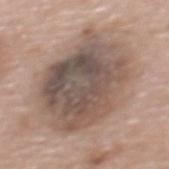The lesion was tiled from a total-body skin photograph and was not biopsied.
The patient is a female approximately 60 years of age.
The lesion is on the upper back.
The lesion's longest dimension is about 9 mm.
A lesion tile, about 15 mm wide, cut from a 3D total-body photograph.